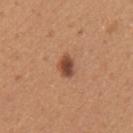A 15 mm close-up tile from a total-body photography series done for melanoma screening.
The subject is a female approximately 45 years of age.
The recorded lesion diameter is about 2.5 mm.
The lesion is on the left upper arm.